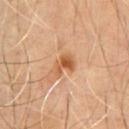Imaged during a routine full-body skin examination; the lesion was not biopsied and no histopathology is available.
A male subject, about 55 years old.
The tile uses cross-polarized illumination.
Automated tile analysis of the lesion measured a footprint of about 5.5 mm², an outline eccentricity of about 0.75 (0 = round, 1 = elongated), and two-axis asymmetry of about 0.35. The software also gave a classifier nevus-likeness of about 50/100 and lesion-presence confidence of about 100/100.
On the chest.
A region of skin cropped from a whole-body photographic capture, roughly 15 mm wide.
The recorded lesion diameter is about 3 mm.